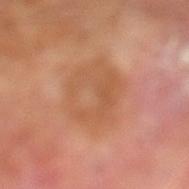biopsy status: total-body-photography surveillance lesion; no biopsy
imaging modality: ~15 mm tile from a whole-body skin photo
tile lighting: cross-polarized illumination
patient: male, in their 70s
body site: the left lower leg
diameter: about 6.5 mm
automated metrics: an eccentricity of roughly 0.65 and two-axis asymmetry of about 0.15; a mean CIELAB color near L≈54 a*≈22 b*≈34, roughly 6 lightness units darker than nearby skin, and a lesion-to-skin contrast of about 5 (normalized; higher = more distinct); a border-irregularity rating of about 2/10 and a color-variation rating of about 4.5/10; an automated nevus-likeness rating near 0 out of 100